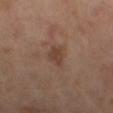Assessment: Imaged during a routine full-body skin examination; the lesion was not biopsied and no histopathology is available. Context: Automated tile analysis of the lesion measured a mean CIELAB color near L≈39 a*≈18 b*≈25, a lesion–skin lightness drop of about 8, and a lesion-to-skin contrast of about 6.5 (normalized; higher = more distinct). A region of skin cropped from a whole-body photographic capture, roughly 15 mm wide. Measured at roughly 2.5 mm in maximum diameter. A male patient about 65 years old.